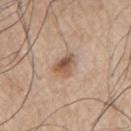notes=catalogued during a skin exam; not biopsied
illumination=white-light
image source=total-body-photography crop, ~15 mm field of view
subject=male, in their mid-70s
anatomic site=the right upper arm